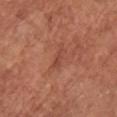The lesion was tiled from a total-body skin photograph and was not biopsied.
A female patient, about 75 years old.
A 15 mm close-up extracted from a 3D total-body photography capture.
On the upper back.
Imaged with white-light lighting.
The lesion's longest dimension is about 3.5 mm.